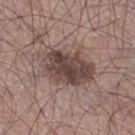Assessment:
The lesion was tiled from a total-body skin photograph and was not biopsied.
Context:
Approximately 6.5 mm at its widest. The tile uses white-light illumination. A close-up tile cropped from a whole-body skin photograph, about 15 mm across. A male subject, about 65 years old. The lesion is located on the right thigh.